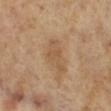The lesion was tiled from a total-body skin photograph and was not biopsied. On the right lower leg. Approximately 4.5 mm at its widest. A 15 mm close-up extracted from a 3D total-body photography capture. The subject is a female aged 68–72.